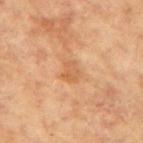Clinical impression:
Captured during whole-body skin photography for melanoma surveillance; the lesion was not biopsied.